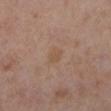Findings:
* workup — no biopsy performed (imaged during a skin exam)
* size — ~2.5 mm (longest diameter)
* acquisition — ~15 mm crop, total-body skin-cancer survey
* subject — female, aged approximately 40
* anatomic site — the left lower leg